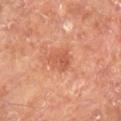| key | value |
|---|---|
| biopsy status | imaged on a skin check; not biopsied |
| patient | male, approximately 70 years of age |
| location | the right lower leg |
| image source | total-body-photography crop, ~15 mm field of view |
| image-analysis metrics | an average lesion color of about L≈55 a*≈29 b*≈34 (CIELAB) and a normalized lesion–skin contrast near 6; a classifier nevus-likeness of about 10/100 |